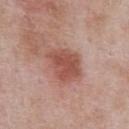{
  "biopsy_status": "not biopsied; imaged during a skin examination",
  "image": {
    "source": "total-body photography crop",
    "field_of_view_mm": 15
  },
  "lesion_size": {
    "long_diameter_mm_approx": 4.5
  },
  "patient": {
    "sex": "male",
    "age_approx": 55
  },
  "site": "front of the torso",
  "lighting": "white-light"
}